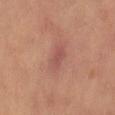Q: Was a biopsy performed?
A: catalogued during a skin exam; not biopsied
Q: Where on the body is the lesion?
A: the right thigh
Q: What are the patient's age and sex?
A: female, aged 33 to 37
Q: What did automated image analysis measure?
A: an average lesion color of about L≈52 a*≈25 b*≈25 (CIELAB) and a lesion-to-skin contrast of about 6 (normalized; higher = more distinct); an automated nevus-likeness rating near 0 out of 100 and a lesion-detection confidence of about 95/100
Q: How was the tile lit?
A: cross-polarized illumination
Q: How was this image acquired?
A: ~15 mm tile from a whole-body skin photo
Q: How large is the lesion?
A: ~4 mm (longest diameter)